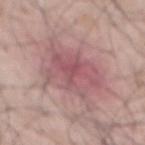Q: Is there a histopathology result?
A: total-body-photography surveillance lesion; no biopsy
Q: What is the anatomic site?
A: the mid back
Q: What are the patient's age and sex?
A: male, aged 53–57
Q: What is the imaging modality?
A: ~15 mm crop, total-body skin-cancer survey
Q: How large is the lesion?
A: ~9.5 mm (longest diameter)